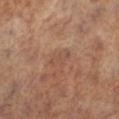notes=total-body-photography surveillance lesion; no biopsy
subject=female, in their mid-60s
body site=the left leg
image source=~15 mm crop, total-body skin-cancer survey